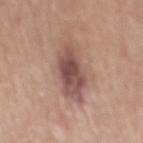Findings:
- biopsy status: imaged on a skin check; not biopsied
- size: ≈6.5 mm
- subject: male, aged 48–52
- image: 15 mm crop, total-body photography
- body site: the mid back